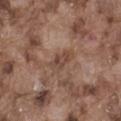Q: Is there a histopathology result?
A: imaged on a skin check; not biopsied
Q: Where on the body is the lesion?
A: the left thigh
Q: Who is the patient?
A: male, approximately 75 years of age
Q: Automated lesion metrics?
A: a mean CIELAB color near L≈44 a*≈18 b*≈26, about 8 CIELAB-L* units darker than the surrounding skin, and a normalized lesion–skin contrast near 6.5; border irregularity of about 3 on a 0–10 scale, a color-variation rating of about 3/10, and peripheral color asymmetry of about 1; a classifier nevus-likeness of about 0/100
Q: What is the imaging modality?
A: ~15 mm crop, total-body skin-cancer survey
Q: How was the tile lit?
A: white-light illumination
Q: What is the lesion's diameter?
A: ~3 mm (longest diameter)